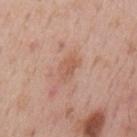biopsy status = no biopsy performed (imaged during a skin exam) | body site = the mid back | diameter = ≈3.5 mm | lighting = white-light illumination | acquisition = 15 mm crop, total-body photography | automated lesion analysis = a footprint of about 5.5 mm², an eccentricity of roughly 0.85, and two-axis asymmetry of about 0.3; a nevus-likeness score of about 0/100 and lesion-presence confidence of about 100/100 | subject = male, approximately 55 years of age.